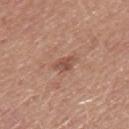| field | value |
|---|---|
| workup | catalogued during a skin exam; not biopsied |
| image source | ~15 mm crop, total-body skin-cancer survey |
| location | the mid back |
| patient | male, aged approximately 50 |
| automated lesion analysis | an area of roughly 4 mm² and a shape-asymmetry score of about 0.35 (0 = symmetric) |
| lesion size | about 3 mm |